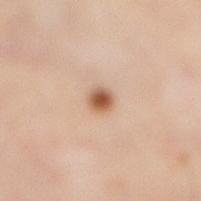The lesion was tiled from a total-body skin photograph and was not biopsied.
About 2 mm across.
Automated image analysis of the tile measured a footprint of about 3 mm², a shape eccentricity near 0.45, and two-axis asymmetry of about 0.15. The software also gave a mean CIELAB color near L≈57 a*≈21 b*≈33, roughly 15 lightness units darker than nearby skin, and a lesion-to-skin contrast of about 10.5 (normalized; higher = more distinct). The analysis additionally found border irregularity of about 1 on a 0–10 scale, internal color variation of about 3.5 on a 0–10 scale, and peripheral color asymmetry of about 1.
A female subject aged 38–42.
Imaged with cross-polarized lighting.
From the right lower leg.
Cropped from a total-body skin-imaging series; the visible field is about 15 mm.